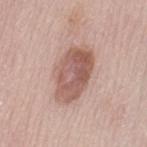Assessment:
No biopsy was performed on this lesion — it was imaged during a full skin examination and was not determined to be concerning.
Image and clinical context:
The subject is a female about 65 years old. The lesion is located on the left thigh. The lesion's longest dimension is about 6.5 mm. A 15 mm crop from a total-body photograph taken for skin-cancer surveillance.